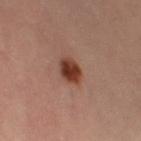* notes — no biopsy performed (imaged during a skin exam)
* diameter — about 3 mm
* TBP lesion metrics — an average lesion color of about L≈37 a*≈24 b*≈27 (CIELAB), roughly 14 lightness units darker than nearby skin, and a normalized border contrast of about 12; a border-irregularity index near 1.5/10, a color-variation rating of about 4/10, and peripheral color asymmetry of about 1.5; a nevus-likeness score of about 100/100
* lighting — cross-polarized
* location — the left thigh
* imaging modality — ~15 mm crop, total-body skin-cancer survey
* subject — female, approximately 35 years of age